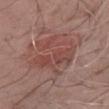No biopsy was performed on this lesion — it was imaged during a full skin examination and was not determined to be concerning.
Located on the chest.
Automated image analysis of the tile measured a footprint of about 22 mm², an eccentricity of roughly 0.5, and a shape-asymmetry score of about 0.4 (0 = symmetric). The software also gave an automated nevus-likeness rating near 20 out of 100.
A roughly 15 mm field-of-view crop from a total-body skin photograph.
A male patient, approximately 80 years of age.